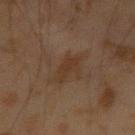notes = imaged on a skin check; not biopsied
image = total-body-photography crop, ~15 mm field of view
anatomic site = the arm
illumination = cross-polarized illumination
subject = male, about 45 years old